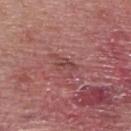| feature | finding |
|---|---|
| biopsy status | catalogued during a skin exam; not biopsied |
| acquisition | total-body-photography crop, ~15 mm field of view |
| patient | male, aged 38 to 42 |
| diameter | ~3 mm (longest diameter) |
| lighting | white-light illumination |
| body site | the upper back |